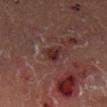Imaged during a routine full-body skin examination; the lesion was not biopsied and no histopathology is available.